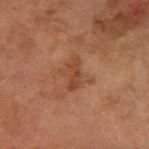workup: total-body-photography surveillance lesion; no biopsy
subject: female, approximately 60 years of age
image-analysis metrics: a footprint of about 4.5 mm² and a shape eccentricity near 0.9; a border-irregularity rating of about 4/10, a within-lesion color-variation index near 1.5/10, and peripheral color asymmetry of about 0.5
anatomic site: the left forearm
imaging modality: total-body-photography crop, ~15 mm field of view
lesion size: ≈3.5 mm